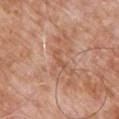The lesion was photographed on a routine skin check and not biopsied; there is no pathology result. Imaged with white-light lighting. The lesion is located on the chest. The recorded lesion diameter is about 2.5 mm. A 15 mm crop from a total-body photograph taken for skin-cancer surveillance. A male subject aged around 80.